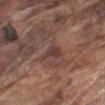biopsy status=catalogued during a skin exam; not biopsied
automated metrics=a mean CIELAB color near L≈39 a*≈18 b*≈21, a lesion–skin lightness drop of about 7, and a normalized lesion–skin contrast near 6.5; an automated nevus-likeness rating near 0 out of 100 and a lesion-detection confidence of about 70/100
acquisition=~15 mm crop, total-body skin-cancer survey
patient=male, aged 73–77
body site=the left upper arm